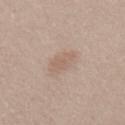Assessment:
The lesion was tiled from a total-body skin photograph and was not biopsied.
Context:
A male subject, aged 28–32. Longest diameter approximately 3 mm. The lesion is on the abdomen. Cropped from a total-body skin-imaging series; the visible field is about 15 mm. An algorithmic analysis of the crop reported a lesion area of about 5 mm², an eccentricity of roughly 0.7, and a symmetry-axis asymmetry near 0.2. The software also gave a normalized border contrast of about 4.5. The software also gave a nevus-likeness score of about 25/100 and a lesion-detection confidence of about 100/100. The tile uses white-light illumination.